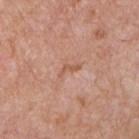  biopsy_status: not biopsied; imaged during a skin examination
  lesion_size:
    long_diameter_mm_approx: 2.5
  patient:
    sex: male
    age_approx: 75
  image:
    source: total-body photography crop
    field_of_view_mm: 15
  automated_metrics:
    area_mm2_approx: 2.0
    eccentricity: 0.95
    shape_asymmetry: 0.7
    border_irregularity_0_10: 7.0
    color_variation_0_10: 0.0
    peripheral_color_asymmetry: 0.0
    nevus_likeness_0_100: 0
  lighting: white-light
  site: front of the torso